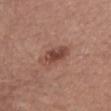| field | value |
|---|---|
| workup | no biopsy performed (imaged during a skin exam) |
| tile lighting | white-light |
| patient | female, about 65 years old |
| site | the front of the torso |
| TBP lesion metrics | a lesion area of about 7 mm², an outline eccentricity of about 0.9 (0 = round, 1 = elongated), and a symmetry-axis asymmetry near 0.15; an average lesion color of about L≈45 a*≈22 b*≈27 (CIELAB), about 11 CIELAB-L* units darker than the surrounding skin, and a lesion-to-skin contrast of about 8 (normalized; higher = more distinct); a border-irregularity index near 2/10, a color-variation rating of about 4/10, and peripheral color asymmetry of about 1; a nevus-likeness score of about 65/100 and lesion-presence confidence of about 100/100 |
| acquisition | ~15 mm tile from a whole-body skin photo |
| lesion diameter | about 4.5 mm |